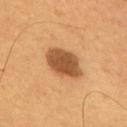Case summary:
* image · total-body-photography crop, ~15 mm field of view
* subject · male, aged around 55
* anatomic site · the back